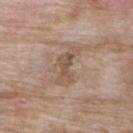No biopsy was performed on this lesion — it was imaged during a full skin examination and was not determined to be concerning. A female subject, roughly 75 years of age. Measured at roughly 4.5 mm in maximum diameter. Imaged with white-light lighting. A close-up tile cropped from a whole-body skin photograph, about 15 mm across. The lesion is on the upper back.